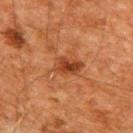biopsy status = catalogued during a skin exam; not biopsied | site = the upper back | patient = male, aged approximately 60 | acquisition = total-body-photography crop, ~15 mm field of view.